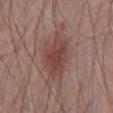Recorded during total-body skin imaging; not selected for excision or biopsy.
Cropped from a total-body skin-imaging series; the visible field is about 15 mm.
The tile uses white-light illumination.
Located on the chest.
The subject is a male about 70 years old.
About 6 mm across.